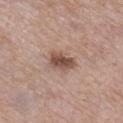Q: What kind of image is this?
A: ~15 mm crop, total-body skin-cancer survey
Q: How large is the lesion?
A: ≈3.5 mm
Q: Lesion location?
A: the right lower leg
Q: Who is the patient?
A: female, about 70 years old